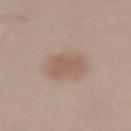<record>
  <biopsy_status>not biopsied; imaged during a skin examination</biopsy_status>
  <lesion_size>
    <long_diameter_mm_approx>3.5</long_diameter_mm_approx>
  </lesion_size>
  <patient>
    <sex>male</sex>
    <age_approx>55</age_approx>
  </patient>
  <image>
    <source>total-body photography crop</source>
    <field_of_view_mm>15</field_of_view_mm>
  </image>
  <lighting>white-light</lighting>
  <site>lower back</site>
</record>